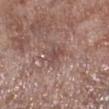Imaged during a routine full-body skin examination; the lesion was not biopsied and no histopathology is available.
A male subject, approximately 55 years of age.
A roughly 15 mm field-of-view crop from a total-body skin photograph.
On the right lower leg.